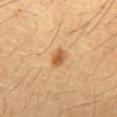Imaged during a routine full-body skin examination; the lesion was not biopsied and no histopathology is available. Longest diameter approximately 2.5 mm. An algorithmic analysis of the crop reported a mean CIELAB color near L≈53 a*≈19 b*≈38, roughly 11 lightness units darker than nearby skin, and a lesion-to-skin contrast of about 8 (normalized; higher = more distinct). The analysis additionally found a border-irregularity index near 1.5/10, a color-variation rating of about 3/10, and peripheral color asymmetry of about 1. Captured under cross-polarized illumination. The lesion is located on the abdomen. A 15 mm crop from a total-body photograph taken for skin-cancer surveillance. A male patient aged 58–62.